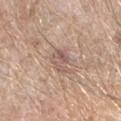Assessment:
Part of a total-body skin-imaging series; this lesion was reviewed on a skin check and was not flagged for biopsy.
Image and clinical context:
From the right forearm. A 15 mm crop from a total-body photograph taken for skin-cancer surveillance. The lesion's longest dimension is about 3 mm. A male subject approximately 80 years of age.